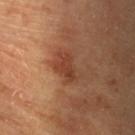A female patient, in their mid-60s. Imaged with cross-polarized lighting. Located on the left forearm. About 4 mm across. A 15 mm close-up extracted from a 3D total-body photography capture. Automated tile analysis of the lesion measured a border-irregularity rating of about 3.5/10, a within-lesion color-variation index near 3/10, and a peripheral color-asymmetry measure near 1.